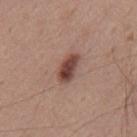notes: catalogued during a skin exam; not biopsied | anatomic site: the mid back | patient: male, aged approximately 55 | acquisition: ~15 mm tile from a whole-body skin photo.